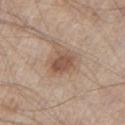Findings:
- image source — ~15 mm crop, total-body skin-cancer survey
- lesion diameter — ≈3 mm
- location — the chest
- illumination — white-light
- subject — male, roughly 80 years of age